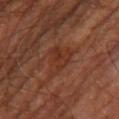{"biopsy_status": "not biopsied; imaged during a skin examination", "lesion_size": {"long_diameter_mm_approx": 4.0}, "image": {"source": "total-body photography crop", "field_of_view_mm": 15}, "site": "right leg", "lighting": "cross-polarized", "patient": {"sex": "male", "age_approx": 60}}